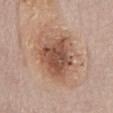biopsy status=imaged on a skin check; not biopsied
subject=male, in their mid-70s
image=total-body-photography crop, ~15 mm field of view
site=the abdomen